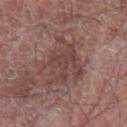acquisition: total-body-photography crop, ~15 mm field of view | site: the left forearm | TBP lesion metrics: a lesion area of about 17 mm², an eccentricity of roughly 0.8, and a shape-asymmetry score of about 0.35 (0 = symmetric); a border-irregularity index near 7.5/10, internal color variation of about 3 on a 0–10 scale, and a peripheral color-asymmetry measure near 1 | size: ≈6.5 mm | patient: male, aged approximately 75 | illumination: white-light illumination.